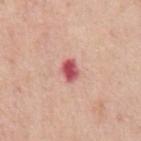On the chest. This image is a 15 mm lesion crop taken from a total-body photograph. A male patient, roughly 75 years of age. The recorded lesion diameter is about 2.5 mm.